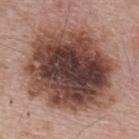| field | value |
|---|---|
| workup | total-body-photography surveillance lesion; no biopsy |
| body site | the upper back |
| lighting | white-light illumination |
| diameter | ~10 mm (longest diameter) |
| patient | male, roughly 65 years of age |
| image | ~15 mm tile from a whole-body skin photo |